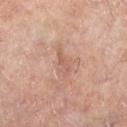* workup — total-body-photography surveillance lesion; no biopsy
* body site — the leg
* patient — male, in their mid-60s
* illumination — cross-polarized
* automated metrics — a footprint of about 3 mm², an outline eccentricity of about 0.9 (0 = round, 1 = elongated), and a symmetry-axis asymmetry near 0.35; an average lesion color of about L≈55 a*≈21 b*≈26 (CIELAB) and a normalized border contrast of about 4.5; a within-lesion color-variation index near 0/10 and a peripheral color-asymmetry measure near 0
* size — ≈3 mm
* image source — ~15 mm tile from a whole-body skin photo